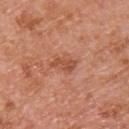Assessment: Recorded during total-body skin imaging; not selected for excision or biopsy. Acquisition and patient details: A 15 mm close-up extracted from a 3D total-body photography capture. A male subject, about 65 years old. On the upper back.